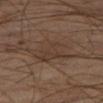Q: Is there a histopathology result?
A: catalogued during a skin exam; not biopsied
Q: Where on the body is the lesion?
A: the leg
Q: Illumination type?
A: cross-polarized
Q: What did automated image analysis measure?
A: a footprint of about 13 mm², a shape eccentricity near 0.35, and two-axis asymmetry of about 0.3; a lesion color around L≈32 a*≈13 b*≈22 in CIELAB and a lesion-to-skin contrast of about 5 (normalized; higher = more distinct); a within-lesion color-variation index near 2.5/10 and radial color variation of about 1; a nevus-likeness score of about 0/100 and lesion-presence confidence of about 90/100
Q: Patient demographics?
A: male, aged 53 to 57
Q: What is the lesion's diameter?
A: ≈4.5 mm
Q: What is the imaging modality?
A: total-body-photography crop, ~15 mm field of view